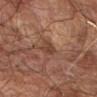The lesion was photographed on a routine skin check and not biopsied; there is no pathology result. The patient is a male in their mid- to late 60s. A 15 mm close-up extracted from a 3D total-body photography capture. Located on the right forearm. Approximately 3 mm at its widest.